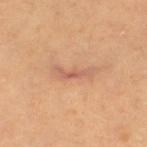Captured during whole-body skin photography for melanoma surveillance; the lesion was not biopsied. The lesion is on the left thigh. This is a cross-polarized tile. A region of skin cropped from a whole-body photographic capture, roughly 15 mm wide. Automated tile analysis of the lesion measured a lesion area of about 4.5 mm², an outline eccentricity of about 0.95 (0 = round, 1 = elongated), and a symmetry-axis asymmetry near 0.55. The software also gave a lesion–skin lightness drop of about 9 and a normalized border contrast of about 6. It also reported a border-irregularity rating of about 6.5/10 and a peripheral color-asymmetry measure near 0. The patient is a female aged approximately 55.